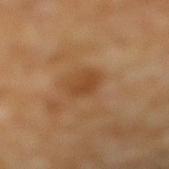Notes:
- follow-up: imaged on a skin check; not biopsied
- automated lesion analysis: an outline eccentricity of about 0.75 (0 = round, 1 = elongated) and two-axis asymmetry of about 0.25; an average lesion color of about L≈41 a*≈20 b*≈35 (CIELAB) and a lesion-to-skin contrast of about 7 (normalized; higher = more distinct)
- image source: ~15 mm crop, total-body skin-cancer survey
- anatomic site: the right lower leg
- illumination: cross-polarized illumination
- patient: male, aged 58–62